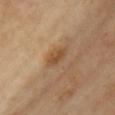notes: imaged on a skin check; not biopsied | size: about 3.5 mm | anatomic site: the chest | TBP lesion metrics: a footprint of about 4.5 mm², an outline eccentricity of about 0.9 (0 = round, 1 = elongated), and a shape-asymmetry score of about 0.2 (0 = symmetric); a lesion color around L≈50 a*≈18 b*≈35 in CIELAB, a lesion–skin lightness drop of about 8, and a normalized lesion–skin contrast near 6.5; a border-irregularity rating of about 2.5/10, internal color variation of about 2.5 on a 0–10 scale, and peripheral color asymmetry of about 1; a nevus-likeness score of about 20/100 and a lesion-detection confidence of about 100/100 | image source: ~15 mm tile from a whole-body skin photo | illumination: cross-polarized illumination | subject: female, roughly 40 years of age.